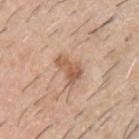• workup · total-body-photography surveillance lesion; no biopsy
• tile lighting · white-light illumination
• image · total-body-photography crop, ~15 mm field of view
• location · the chest
• subject · male, aged approximately 60
• automated lesion analysis · a border-irregularity rating of about 4/10, a color-variation rating of about 3.5/10, and a peripheral color-asymmetry measure near 1; a nevus-likeness score of about 50/100 and a lesion-detection confidence of about 100/100
• lesion diameter · ≈3.5 mm